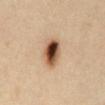Captured during whole-body skin photography for melanoma surveillance; the lesion was not biopsied.
The total-body-photography lesion software estimated a lesion color around L≈51 a*≈20 b*≈33 in CIELAB, about 21 CIELAB-L* units darker than the surrounding skin, and a normalized lesion–skin contrast near 13.5. It also reported a border-irregularity rating of about 2/10, internal color variation of about 10 on a 0–10 scale, and radial color variation of about 4.5.
Imaged with cross-polarized lighting.
A lesion tile, about 15 mm wide, cut from a 3D total-body photograph.
On the abdomen.
Longest diameter approximately 4 mm.
The subject is a male aged 38 to 42.